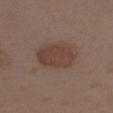Q: What are the patient's age and sex?
A: female, in their mid-30s
Q: What is the imaging modality?
A: ~15 mm crop, total-body skin-cancer survey
Q: Illumination type?
A: white-light illumination
Q: What is the anatomic site?
A: the left upper arm
Q: What did automated image analysis measure?
A: a footprint of about 13 mm² and two-axis asymmetry of about 0.15